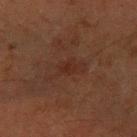imaging modality = ~15 mm crop, total-body skin-cancer survey
tile lighting = cross-polarized illumination
size = about 5 mm
location = the left forearm
subject = male, aged around 65